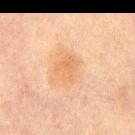<tbp_lesion>
  <biopsy_status>not biopsied; imaged during a skin examination</biopsy_status>
  <patient>
    <sex>male</sex>
    <age_approx>65</age_approx>
  </patient>
  <lighting>cross-polarized</lighting>
  <image>
    <source>total-body photography crop</source>
    <field_of_view_mm>15</field_of_view_mm>
  </image>
  <lesion_size>
    <long_diameter_mm_approx>3.0</long_diameter_mm_approx>
  </lesion_size>
  <site>mid back</site>
</tbp_lesion>